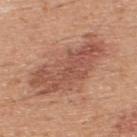  patient:
    sex: male
    age_approx: 45
  automated_metrics:
    cielab_L: 53
    cielab_a: 24
    cielab_b: 29
    vs_skin_darker_L: 10.0
    vs_skin_contrast_norm: 7.5
    border_irregularity_0_10: 5.5
    color_variation_0_10: 4.5
    peripheral_color_asymmetry: 1.5
  lesion_size:
    long_diameter_mm_approx: 9.0
  image:
    source: total-body photography crop
    field_of_view_mm: 15
  site: back
  lighting: white-light